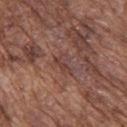Captured under white-light illumination.
The patient is a male in their mid-70s.
Located on the upper back.
A lesion tile, about 15 mm wide, cut from a 3D total-body photograph.